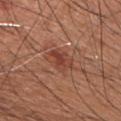  biopsy_status: not biopsied; imaged during a skin examination
  lighting: white-light
  image:
    source: total-body photography crop
    field_of_view_mm: 15
  patient:
    sex: male
    age_approx: 60
  site: chest